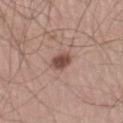Assessment: The lesion was tiled from a total-body skin photograph and was not biopsied. Image and clinical context: A lesion tile, about 15 mm wide, cut from a 3D total-body photograph. The lesion is located on the leg. The patient is a male about 30 years old.